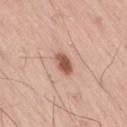The lesion was photographed on a routine skin check and not biopsied; there is no pathology result.
The patient is a male aged around 55.
The tile uses white-light illumination.
Measured at roughly 3 mm in maximum diameter.
A 15 mm crop from a total-body photograph taken for skin-cancer surveillance.
The total-body-photography lesion software estimated an average lesion color of about L≈55 a*≈23 b*≈29 (CIELAB), a lesion–skin lightness drop of about 15, and a lesion-to-skin contrast of about 9.5 (normalized; higher = more distinct). The analysis additionally found a nevus-likeness score of about 95/100 and a detector confidence of about 100 out of 100 that the crop contains a lesion.
From the right thigh.